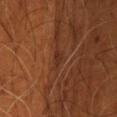patient: male, aged 53 to 57 | tile lighting: cross-polarized | acquisition: ~15 mm tile from a whole-body skin photo | TBP lesion metrics: an area of roughly 2.5 mm², a shape eccentricity near 0.9, and two-axis asymmetry of about 0.35; roughly 5 lightness units darker than nearby skin and a lesion-to-skin contrast of about 6 (normalized; higher = more distinct) | site: the head or neck.